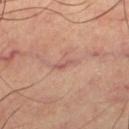follow-up=catalogued during a skin exam; not biopsied
acquisition=~15 mm tile from a whole-body skin photo
site=the left thigh
subject=male, about 65 years old
illumination=cross-polarized